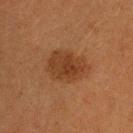{
  "biopsy_status": "not biopsied; imaged during a skin examination",
  "site": "head or neck",
  "image": {
    "source": "total-body photography crop",
    "field_of_view_mm": 15
  },
  "lighting": "cross-polarized",
  "lesion_size": {
    "long_diameter_mm_approx": 5.0
  },
  "patient": {
    "sex": "female",
    "age_approx": 40
  }
}